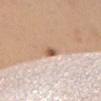Imaged during a routine full-body skin examination; the lesion was not biopsied and no histopathology is available.
A female patient, roughly 45 years of age.
A close-up tile cropped from a whole-body skin photograph, about 15 mm across.
The recorded lesion diameter is about 3 mm.
The total-body-photography lesion software estimated a lesion color around L≈61 a*≈18 b*≈32 in CIELAB, about 11 CIELAB-L* units darker than the surrounding skin, and a normalized lesion–skin contrast near 7.5. And it measured an automated nevus-likeness rating near 95 out of 100 and a lesion-detection confidence of about 100/100.
On the chest.